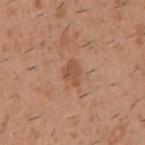Clinical impression: Part of a total-body skin-imaging series; this lesion was reviewed on a skin check and was not flagged for biopsy. Acquisition and patient details: Approximately 3 mm at its widest. The patient is a male about 40 years old. Located on the right upper arm. Cropped from a whole-body photographic skin survey; the tile spans about 15 mm. Automated tile analysis of the lesion measured a mean CIELAB color near L≈51 a*≈22 b*≈32 and a normalized border contrast of about 6. It also reported a border-irregularity index near 3.5/10 and peripheral color asymmetry of about 0.5. The analysis additionally found an automated nevus-likeness rating near 0 out of 100. This is a white-light tile.